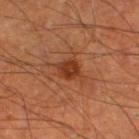{"biopsy_status": "not biopsied; imaged during a skin examination", "site": "left lower leg", "patient": {"sex": "male", "age_approx": 65}, "lesion_size": {"long_diameter_mm_approx": 2.5}, "lighting": "cross-polarized", "image": {"source": "total-body photography crop", "field_of_view_mm": 15}}